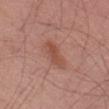Impression: Part of a total-body skin-imaging series; this lesion was reviewed on a skin check and was not flagged for biopsy. Image and clinical context: A male patient, aged 53–57. From the left thigh. A lesion tile, about 15 mm wide, cut from a 3D total-body photograph. About 4 mm across. This is a white-light tile.